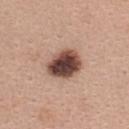biopsy_status: not biopsied; imaged during a skin examination
patient:
  sex: female
  age_approx: 40
image:
  source: total-body photography crop
  field_of_view_mm: 15
site: upper back